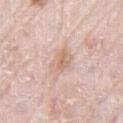{
  "biopsy_status": "not biopsied; imaged during a skin examination",
  "patient": {
    "sex": "male",
    "age_approx": 80
  },
  "image": {
    "source": "total-body photography crop",
    "field_of_view_mm": 15
  },
  "site": "right thigh",
  "lesion_size": {
    "long_diameter_mm_approx": 3.5
  }
}